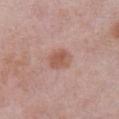| key | value |
|---|---|
| follow-up | imaged on a skin check; not biopsied |
| anatomic site | the abdomen |
| subject | male, in their mid-50s |
| diameter | ≈3 mm |
| image | ~15 mm crop, total-body skin-cancer survey |
| tile lighting | white-light |